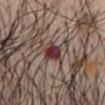Notes:
* workup · imaged on a skin check; not biopsied
* imaging modality · 15 mm crop, total-body photography
* size · ~2.5 mm (longest diameter)
* body site · the chest
* patient · male, approximately 55 years of age
* illumination · white-light